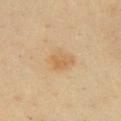Case summary:
– workup · no biopsy performed (imaged during a skin exam)
– size · ~3.5 mm (longest diameter)
– lighting · cross-polarized
– image-analysis metrics · a footprint of about 8 mm² and a shape eccentricity near 0.55; a border-irregularity rating of about 2/10, a color-variation rating of about 3/10, and radial color variation of about 1; a lesion-detection confidence of about 100/100
– subject · female, aged 58 to 62
– image · ~15 mm crop, total-body skin-cancer survey
– location · the front of the torso